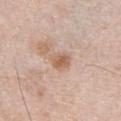This lesion was catalogued during total-body skin photography and was not selected for biopsy. A roughly 15 mm field-of-view crop from a total-body skin photograph. The recorded lesion diameter is about 2.5 mm. On the chest. A male subject about 80 years old. Captured under white-light illumination.